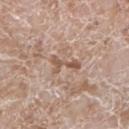The lesion is located on the left lower leg.
This image is a 15 mm lesion crop taken from a total-body photograph.
Measured at roughly 4 mm in maximum diameter.
The total-body-photography lesion software estimated an eccentricity of roughly 0.9. The analysis additionally found a mean CIELAB color near L≈55 a*≈18 b*≈28, a lesion–skin lightness drop of about 10, and a normalized border contrast of about 7.5. It also reported internal color variation of about 0 on a 0–10 scale and peripheral color asymmetry of about 0. The analysis additionally found a detector confidence of about 75 out of 100 that the crop contains a lesion.
A male patient, aged around 60.
The tile uses white-light illumination.